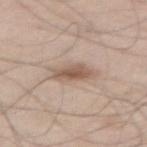follow-up: no biopsy performed (imaged during a skin exam)
diameter: ≈4 mm
patient: male, aged around 60
automated metrics: an area of roughly 7 mm², a shape eccentricity near 0.85, and two-axis asymmetry of about 0.25; a mean CIELAB color near L≈57 a*≈16 b*≈27, about 11 CIELAB-L* units darker than the surrounding skin, and a normalized lesion–skin contrast near 7.5; a border-irregularity index near 3/10 and a peripheral color-asymmetry measure near 1; a classifier nevus-likeness of about 85/100 and a detector confidence of about 100 out of 100 that the crop contains a lesion
lighting: white-light illumination
image: ~15 mm crop, total-body skin-cancer survey
body site: the right thigh